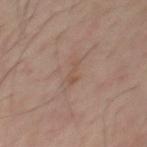The lesion was tiled from a total-body skin photograph and was not biopsied.
On the chest.
A 15 mm close-up tile from a total-body photography series done for melanoma screening.
The patient is a male roughly 65 years of age.
The recorded lesion diameter is about 3 mm.